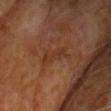The lesion was photographed on a routine skin check and not biopsied; there is no pathology result.
About 4 mm across.
A 15 mm crop from a total-body photograph taken for skin-cancer surveillance.
The lesion is located on the back.
A male subject, in their 70s.
Imaged with cross-polarized lighting.